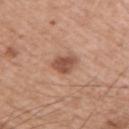{
  "biopsy_status": "not biopsied; imaged during a skin examination",
  "image": {
    "source": "total-body photography crop",
    "field_of_view_mm": 15
  },
  "lesion_size": {
    "long_diameter_mm_approx": 3.0
  },
  "patient": {
    "sex": "male",
    "age_approx": 55
  },
  "lighting": "white-light",
  "site": "right upper arm"
}